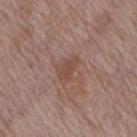• follow-up: total-body-photography surveillance lesion; no biopsy
• subject: male, aged around 70
• anatomic site: the mid back
• image: 15 mm crop, total-body photography